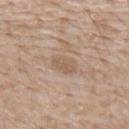Q: Was this lesion biopsied?
A: catalogued during a skin exam; not biopsied
Q: Who is the patient?
A: male, about 65 years old
Q: How was this image acquired?
A: ~15 mm crop, total-body skin-cancer survey
Q: What did automated image analysis measure?
A: a border-irregularity rating of about 1.5/10 and a within-lesion color-variation index near 2/10
Q: What is the anatomic site?
A: the mid back
Q: What is the lesion's diameter?
A: about 3.5 mm